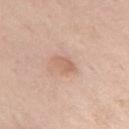This lesion was catalogued during total-body skin photography and was not selected for biopsy. Cropped from a total-body skin-imaging series; the visible field is about 15 mm. Measured at roughly 3 mm in maximum diameter. The patient is a male in their 70s. From the upper back. This is a white-light tile.